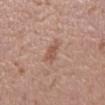This lesion was catalogued during total-body skin photography and was not selected for biopsy. Automated tile analysis of the lesion measured a footprint of about 4 mm² and a shape-asymmetry score of about 0.3 (0 = symmetric). The analysis additionally found a border-irregularity index near 3/10 and a peripheral color-asymmetry measure near 0.5. From the right lower leg. Measured at roughly 2.5 mm in maximum diameter. A female subject aged 28–32. A 15 mm close-up tile from a total-body photography series done for melanoma screening. Imaged with white-light lighting.